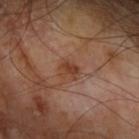Imaged during a routine full-body skin examination; the lesion was not biopsied and no histopathology is available. From the left upper arm. Automated image analysis of the tile measured a footprint of about 4 mm² and a shape eccentricity near 0.55. It also reported a lesion color around L≈41 a*≈22 b*≈31 in CIELAB and a lesion–skin lightness drop of about 8. It also reported a border-irregularity index near 3/10, internal color variation of about 2 on a 0–10 scale, and peripheral color asymmetry of about 0.5. The analysis additionally found an automated nevus-likeness rating near 40 out of 100 and lesion-presence confidence of about 100/100. A region of skin cropped from a whole-body photographic capture, roughly 15 mm wide. Imaged with cross-polarized lighting. A male subject aged around 65.